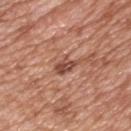Assessment:
The lesion was tiled from a total-body skin photograph and was not biopsied.
Clinical summary:
A 15 mm crop from a total-body photograph taken for skin-cancer surveillance. On the chest. The recorded lesion diameter is about 3.5 mm. A male patient, aged around 50.